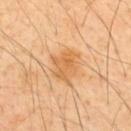notes: total-body-photography surveillance lesion; no biopsy | lesion size: ≈4.5 mm | subject: male, in their 50s | image: ~15 mm crop, total-body skin-cancer survey | body site: the upper back.